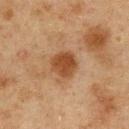{"biopsy_status": "not biopsied; imaged during a skin examination", "lighting": "cross-polarized", "lesion_size": {"long_diameter_mm_approx": 3.5}, "automated_metrics": {"area_mm2_approx": 8.0, "eccentricity": 0.15, "cielab_L": 38, "cielab_a": 19, "cielab_b": 31, "vs_skin_darker_L": 10.0, "border_irregularity_0_10": 2.0, "color_variation_0_10": 2.5, "peripheral_color_asymmetry": 1.0}, "patient": {"sex": "male", "age_approx": 75}, "image": {"source": "total-body photography crop", "field_of_view_mm": 15}, "site": "chest"}